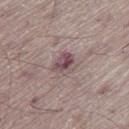<lesion>
<biopsy_status>not biopsied; imaged during a skin examination</biopsy_status>
<lesion_size>
  <long_diameter_mm_approx>3.0</long_diameter_mm_approx>
</lesion_size>
<lighting>white-light</lighting>
<site>right thigh</site>
<image>
  <source>total-body photography crop</source>
  <field_of_view_mm>15</field_of_view_mm>
</image>
<automated_metrics>
  <nevus_likeness_0_100>0</nevus_likeness_0_100>
  <lesion_detection_confidence_0_100>85</lesion_detection_confidence_0_100>
</automated_metrics>
<patient>
  <sex>male</sex>
  <age_approx>70</age_approx>
</patient>
</lesion>